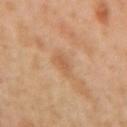Automated image analysis of the tile measured a lesion area of about 5 mm² and a symmetry-axis asymmetry near 0.3.
The tile uses cross-polarized illumination.
The subject is a female aged 38 to 42.
The lesion's longest dimension is about 2.5 mm.
A 15 mm close-up tile from a total-body photography series done for melanoma screening.
From the left forearm.